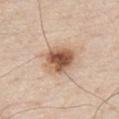Clinical impression: The lesion was photographed on a routine skin check and not biopsied; there is no pathology result. Acquisition and patient details: Automated tile analysis of the lesion measured an area of roughly 13 mm², an eccentricity of roughly 0.5, and a symmetry-axis asymmetry near 0.2. The software also gave an average lesion color of about L≈58 a*≈20 b*≈31 (CIELAB), a lesion–skin lightness drop of about 16, and a normalized border contrast of about 10. It also reported a border-irregularity index near 2.5/10 and a within-lesion color-variation index near 8.5/10. On the chest. A male patient, in their 80s. Longest diameter approximately 4.5 mm. The tile uses white-light illumination. This image is a 15 mm lesion crop taken from a total-body photograph.